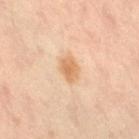Imaged during a routine full-body skin examination; the lesion was not biopsied and no histopathology is available. A region of skin cropped from a whole-body photographic capture, roughly 15 mm wide. On the right thigh. The patient is a female aged approximately 40.